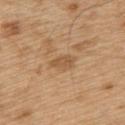Notes:
• workup: imaged on a skin check; not biopsied
• body site: the upper back
• illumination: white-light
• size: ~3 mm (longest diameter)
• image source: ~15 mm crop, total-body skin-cancer survey
• patient: male, about 70 years old